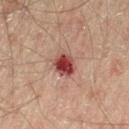No biopsy was performed on this lesion — it was imaged during a full skin examination and was not determined to be concerning. The lesion is located on the left thigh. The lesion-visualizer software estimated a lesion–skin lightness drop of about 14 and a lesion-to-skin contrast of about 11.5 (normalized; higher = more distinct). It also reported a border-irregularity rating of about 2/10, a within-lesion color-variation index near 5.5/10, and radial color variation of about 1.5. The analysis additionally found an automated nevus-likeness rating near 0 out of 100. The subject is a male about 45 years old. The tile uses cross-polarized illumination. The recorded lesion diameter is about 3 mm. A region of skin cropped from a whole-body photographic capture, roughly 15 mm wide.